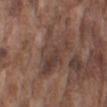Q: Is there a histopathology result?
A: imaged on a skin check; not biopsied
Q: Automated lesion metrics?
A: an automated nevus-likeness rating near 0 out of 100 and lesion-presence confidence of about 50/100
Q: Lesion location?
A: the right upper arm
Q: Lesion size?
A: ≈5.5 mm
Q: What is the imaging modality?
A: ~15 mm crop, total-body skin-cancer survey
Q: Patient demographics?
A: male, in their mid- to late 70s